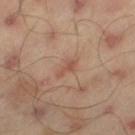{
  "biopsy_status": "not biopsied; imaged during a skin examination",
  "patient": {
    "sex": "male",
    "age_approx": 45
  },
  "automated_metrics": {
    "area_mm2_approx": 2.5,
    "eccentricity": 0.9,
    "shape_asymmetry": 0.5,
    "border_irregularity_0_10": 5.0,
    "color_variation_0_10": 0.0
  },
  "image": {
    "source": "total-body photography crop",
    "field_of_view_mm": 15
  },
  "lesion_size": {
    "long_diameter_mm_approx": 2.5
  },
  "site": "leg"
}